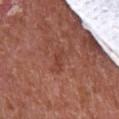Impression:
No biopsy was performed on this lesion — it was imaged during a full skin examination and was not determined to be concerning.
Image and clinical context:
The lesion is on the chest. A roughly 15 mm field-of-view crop from a total-body skin photograph. Longest diameter approximately 3.5 mm. A male subject aged around 65. The tile uses white-light illumination. The lesion-visualizer software estimated a lesion area of about 3 mm², an outline eccentricity of about 0.95 (0 = round, 1 = elongated), and two-axis asymmetry of about 0.45. It also reported roughly 6 lightness units darker than nearby skin and a lesion-to-skin contrast of about 5 (normalized; higher = more distinct). The analysis additionally found a border-irregularity index near 5/10 and a peripheral color-asymmetry measure near 0.